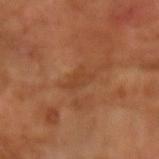The lesion was tiled from a total-body skin photograph and was not biopsied. The subject is a male aged approximately 65. Automated tile analysis of the lesion measured a lesion area of about 4 mm². The analysis additionally found an automated nevus-likeness rating near 0 out of 100 and a lesion-detection confidence of about 100/100. The tile uses cross-polarized illumination. Cropped from a total-body skin-imaging series; the visible field is about 15 mm.